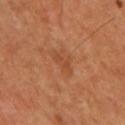On the mid back. A lesion tile, about 15 mm wide, cut from a 3D total-body photograph. A male patient in their 60s.